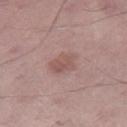  biopsy_status: not biopsied; imaged during a skin examination
  site: right thigh
  automated_metrics:
    cielab_L: 54
    cielab_a: 20
    cielab_b: 21
    vs_skin_contrast_norm: 5.5
    color_variation_0_10: 2.5
    peripheral_color_asymmetry: 1.0
  lesion_size:
    long_diameter_mm_approx: 3.5
  lighting: white-light
  image:
    source: total-body photography crop
    field_of_view_mm: 15
  patient:
    sex: male
    age_approx: 55